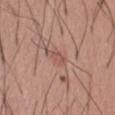| feature | finding |
|---|---|
| follow-up | no biopsy performed (imaged during a skin exam) |
| location | the abdomen |
| lighting | white-light illumination |
| image-analysis metrics | a border-irregularity index near 4.5/10, internal color variation of about 2 on a 0–10 scale, and radial color variation of about 0.5; a detector confidence of about 100 out of 100 that the crop contains a lesion |
| imaging modality | ~15 mm crop, total-body skin-cancer survey |
| patient | male, aged 53–57 |